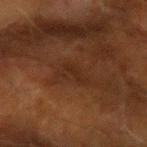Impression: Captured during whole-body skin photography for melanoma surveillance; the lesion was not biopsied. Context: Automated tile analysis of the lesion measured a lesion area of about 4 mm², a shape eccentricity near 0.85, and a symmetry-axis asymmetry near 0.5. The software also gave a mean CIELAB color near L≈21 a*≈16 b*≈23, about 4 CIELAB-L* units darker than the surrounding skin, and a normalized border contrast of about 5.5. And it measured a border-irregularity rating of about 7/10, a color-variation rating of about 0/10, and a peripheral color-asymmetry measure near 0. Cropped from a total-body skin-imaging series; the visible field is about 15 mm. The lesion is located on the right forearm. A male patient, roughly 65 years of age. The tile uses cross-polarized illumination.